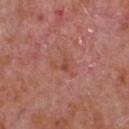The lesion was photographed on a routine skin check and not biopsied; there is no pathology result. A 15 mm close-up extracted from a 3D total-body photography capture. The patient is a male aged approximately 65. Located on the chest.